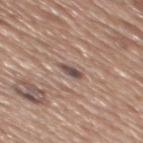Recorded during total-body skin imaging; not selected for excision or biopsy. This is a white-light tile. The patient is a male about 65 years old. A 15 mm crop from a total-body photograph taken for skin-cancer surveillance. The lesion's longest dimension is about 2.5 mm. The lesion is located on the mid back.